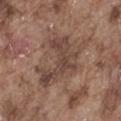Case summary:
* follow-up · total-body-photography surveillance lesion; no biopsy
* site · the abdomen
* TBP lesion metrics · a border-irregularity index near 9/10, a color-variation rating of about 3.5/10, and a peripheral color-asymmetry measure near 1; a nevus-likeness score of about 0/100 and a lesion-detection confidence of about 90/100
* lighting · white-light
* subject · male, aged 73–77
* image source · 15 mm crop, total-body photography